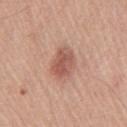Q: Was a biopsy performed?
A: no biopsy performed (imaged during a skin exam)
Q: What is the imaging modality?
A: total-body-photography crop, ~15 mm field of view
Q: Illumination type?
A: white-light
Q: Who is the patient?
A: male, aged 53–57
Q: Lesion size?
A: ~3.5 mm (longest diameter)
Q: Lesion location?
A: the chest